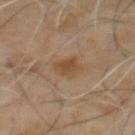| feature | finding |
|---|---|
| follow-up | catalogued during a skin exam; not biopsied |
| anatomic site | the mid back |
| diameter | about 3.5 mm |
| image source | total-body-photography crop, ~15 mm field of view |
| automated lesion analysis | a lesion area of about 6 mm², an outline eccentricity of about 0.75 (0 = round, 1 = elongated), and a shape-asymmetry score of about 0.2 (0 = symmetric); a detector confidence of about 100 out of 100 that the crop contains a lesion |
| subject | male, aged approximately 60 |